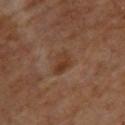This lesion was catalogued during total-body skin photography and was not selected for biopsy. A 15 mm close-up tile from a total-body photography series done for melanoma screening. About 3 mm across. Located on the upper back. Automated image analysis of the tile measured a border-irregularity index near 2.5/10. A female patient, in their mid-60s.